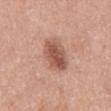Findings:
* follow-up: imaged on a skin check; not biopsied
* illumination: white-light
* subject: female, aged 38 to 42
* lesion diameter: ≈5 mm
* acquisition: 15 mm crop, total-body photography
* anatomic site: the mid back
* automated metrics: a shape eccentricity near 0.85 and a symmetry-axis asymmetry near 0.25; a border-irregularity index near 2.5/10 and a peripheral color-asymmetry measure near 2; a classifier nevus-likeness of about 80/100 and a lesion-detection confidence of about 100/100